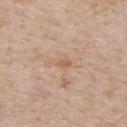This lesion was catalogued during total-body skin photography and was not selected for biopsy.
The lesion is located on the upper back.
A male patient approximately 60 years of age.
A 15 mm close-up tile from a total-body photography series done for melanoma screening.